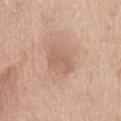{"biopsy_status": "not biopsied; imaged during a skin examination", "patient": {"sex": "male", "age_approx": 65}, "image": {"source": "total-body photography crop", "field_of_view_mm": 15}, "site": "chest"}